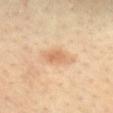This lesion was catalogued during total-body skin photography and was not selected for biopsy.
A female patient roughly 40 years of age.
A lesion tile, about 15 mm wide, cut from a 3D total-body photograph.
Measured at roughly 2.5 mm in maximum diameter.
The lesion is located on the chest.
The tile uses cross-polarized illumination.